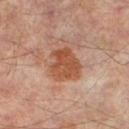  biopsy_status: not biopsied; imaged during a skin examination
  site: left thigh
  image:
    source: total-body photography crop
    field_of_view_mm: 15
  patient:
    sex: male
    age_approx: 65